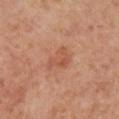Clinical impression:
Recorded during total-body skin imaging; not selected for excision or biopsy.
Context:
The recorded lesion diameter is about 3.5 mm. This is a cross-polarized tile. The total-body-photography lesion software estimated an average lesion color of about L≈55 a*≈26 b*≈33 (CIELAB) and roughly 7 lightness units darker than nearby skin. It also reported a border-irregularity index near 4.5/10, internal color variation of about 3 on a 0–10 scale, and radial color variation of about 1. The analysis additionally found an automated nevus-likeness rating near 0 out of 100 and a lesion-detection confidence of about 100/100. A roughly 15 mm field-of-view crop from a total-body skin photograph. A female patient, in their mid-50s. Located on the leg.